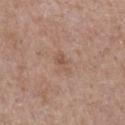Imaged during a routine full-body skin examination; the lesion was not biopsied and no histopathology is available.
A female subject, roughly 40 years of age.
Captured under white-light illumination.
Located on the left forearm.
A 15 mm close-up extracted from a 3D total-body photography capture.